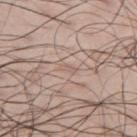Image and clinical context:
Longest diameter approximately 2 mm. Cropped from a total-body skin-imaging series; the visible field is about 15 mm. From the left upper arm. The patient is a male approximately 45 years of age.